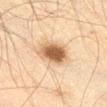{"biopsy_status": "not biopsied; imaged during a skin examination", "patient": {"sex": "male", "age_approx": 60}, "lesion_size": {"long_diameter_mm_approx": 3.5}, "site": "front of the torso", "image": {"source": "total-body photography crop", "field_of_view_mm": 15}, "automated_metrics": {"area_mm2_approx": 10.0, "eccentricity": 0.4, "shape_asymmetry": 0.15, "cielab_L": 47, "cielab_a": 16, "cielab_b": 29, "vs_skin_darker_L": 14.0, "vs_skin_contrast_norm": 10.5}}